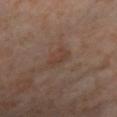Assessment: Recorded during total-body skin imaging; not selected for excision or biopsy. Acquisition and patient details: The patient is a female approximately 55 years of age. A region of skin cropped from a whole-body photographic capture, roughly 15 mm wide. Automated tile analysis of the lesion measured an average lesion color of about L≈40 a*≈17 b*≈25 (CIELAB) and a normalized border contrast of about 5.5. The lesion is on the leg. Imaged with cross-polarized lighting.